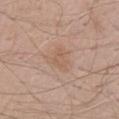biopsy_status: not biopsied; imaged during a skin examination
site: back
lesion_size:
  long_diameter_mm_approx: 3.5
image:
  source: total-body photography crop
  field_of_view_mm: 15
patient:
  sex: male
  age_approx: 60